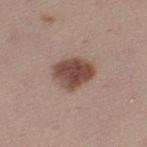Clinical impression:
Imaged during a routine full-body skin examination; the lesion was not biopsied and no histopathology is available.
Image and clinical context:
A close-up tile cropped from a whole-body skin photograph, about 15 mm across. A female patient about 45 years old. On the left thigh.